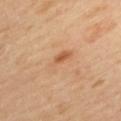follow-up: catalogued during a skin exam; not biopsied | subject: female, aged 38 to 42 | location: the upper back | image source: 15 mm crop, total-body photography.